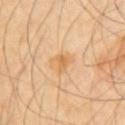workup: no biopsy performed (imaged during a skin exam) | body site: the front of the torso | lighting: cross-polarized illumination | automated metrics: an area of roughly 4.5 mm² and a shape eccentricity near 0.45; an average lesion color of about L≈69 a*≈20 b*≈44 (CIELAB), a lesion–skin lightness drop of about 8, and a lesion-to-skin contrast of about 6 (normalized; higher = more distinct); a classifier nevus-likeness of about 0/100 and a lesion-detection confidence of about 100/100 | size: ≈3 mm | image source: total-body-photography crop, ~15 mm field of view.